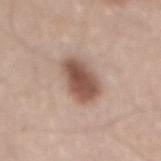| feature | finding |
|---|---|
| subject | male, approximately 45 years of age |
| image-analysis metrics | an area of roughly 12 mm², an outline eccentricity of about 0.75 (0 = round, 1 = elongated), and two-axis asymmetry of about 0.15; a mean CIELAB color near L≈52 a*≈19 b*≈25, roughly 15 lightness units darker than nearby skin, and a lesion-to-skin contrast of about 10.5 (normalized; higher = more distinct); an automated nevus-likeness rating near 95 out of 100 and a detector confidence of about 100 out of 100 that the crop contains a lesion |
| lesion diameter | ≈4.5 mm |
| image | 15 mm crop, total-body photography |
| location | the mid back |